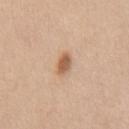Imaged during a routine full-body skin examination; the lesion was not biopsied and no histopathology is available.
Longest diameter approximately 2.5 mm.
Automated tile analysis of the lesion measured a shape eccentricity near 0.75 and a shape-asymmetry score of about 0.2 (0 = symmetric). And it measured a lesion color around L≈59 a*≈20 b*≈34 in CIELAB, about 12 CIELAB-L* units darker than the surrounding skin, and a lesion-to-skin contrast of about 8.5 (normalized; higher = more distinct).
A male patient, approximately 40 years of age.
The lesion is on the chest.
A 15 mm crop from a total-body photograph taken for skin-cancer surveillance.
Captured under white-light illumination.